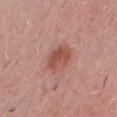Clinical impression: Imaged during a routine full-body skin examination; the lesion was not biopsied and no histopathology is available. Clinical summary: An algorithmic analysis of the crop reported a footprint of about 7.5 mm² and two-axis asymmetry of about 0.2. The software also gave a lesion color around L≈51 a*≈26 b*≈27 in CIELAB and a normalized border contrast of about 7.5. The analysis additionally found an automated nevus-likeness rating near 80 out of 100 and a detector confidence of about 100 out of 100 that the crop contains a lesion. A lesion tile, about 15 mm wide, cut from a 3D total-body photograph. The subject is a male in their 50s. The lesion is located on the chest.